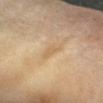Background: Automated tile analysis of the lesion measured a lesion color around L≈57 a*≈14 b*≈35 in CIELAB and a normalized border contrast of about 4.5. The analysis additionally found a detector confidence of about 100 out of 100 that the crop contains a lesion. A female subject approximately 60 years of age. The lesion is on the left forearm. A 15 mm crop from a total-body photograph taken for skin-cancer surveillance. Measured at roughly 3 mm in maximum diameter.